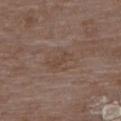This lesion was catalogued during total-body skin photography and was not selected for biopsy.
A 15 mm close-up extracted from a 3D total-body photography capture.
Measured at roughly 3 mm in maximum diameter.
Automated image analysis of the tile measured a lesion area of about 3.5 mm², a shape eccentricity near 0.9, and a shape-asymmetry score of about 0.3 (0 = symmetric). And it measured roughly 5 lightness units darker than nearby skin and a normalized lesion–skin contrast near 5.
The lesion is located on the upper back.
A female patient aged 68 to 72.